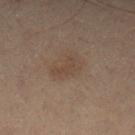Impression:
The lesion was photographed on a routine skin check and not biopsied; there is no pathology result.
Context:
Located on the left lower leg. Measured at roughly 3.5 mm in maximum diameter. Automated image analysis of the tile measured an area of roughly 4.5 mm², an eccentricity of roughly 0.9, and a shape-asymmetry score of about 0.45 (0 = symmetric). And it measured a detector confidence of about 100 out of 100 that the crop contains a lesion. A male subject in their mid-50s. This is a cross-polarized tile. This image is a 15 mm lesion crop taken from a total-body photograph.